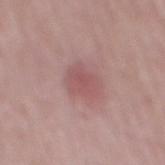Recorded during total-body skin imaging; not selected for excision or biopsy. Imaged with white-light lighting. A 15 mm crop from a total-body photograph taken for skin-cancer surveillance. The patient is a male roughly 65 years of age. The recorded lesion diameter is about 3.5 mm. On the mid back.